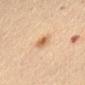{
  "biopsy_status": "not biopsied; imaged during a skin examination",
  "lighting": "cross-polarized",
  "automated_metrics": {
    "border_irregularity_0_10": 2.0,
    "peripheral_color_asymmetry": 1.0
  },
  "image": {
    "source": "total-body photography crop",
    "field_of_view_mm": 15
  },
  "site": "lower back",
  "patient": {
    "sex": "female",
    "age_approx": 70
  }
}